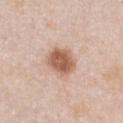| field | value |
|---|---|
| follow-up | total-body-photography surveillance lesion; no biopsy |